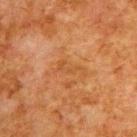Findings:
* follow-up — catalogued during a skin exam; not biopsied
* location — the upper back
* imaging modality — ~15 mm crop, total-body skin-cancer survey
* lighting — cross-polarized
* subject — male, aged 78 to 82